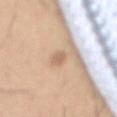Assessment: Part of a total-body skin-imaging series; this lesion was reviewed on a skin check and was not flagged for biopsy. Background: A roughly 15 mm field-of-view crop from a total-body skin photograph. The patient is a male in their mid- to late 50s. The lesion is located on the back.